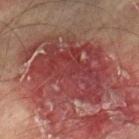biopsy status = total-body-photography surveillance lesion; no biopsy | lighting = cross-polarized | image-analysis metrics = a lesion area of about 60 mm², a shape eccentricity near 0.7, and two-axis asymmetry of about 0.25; a border-irregularity index near 5.5/10, internal color variation of about 6.5 on a 0–10 scale, and a peripheral color-asymmetry measure near 2; a detector confidence of about 85 out of 100 that the crop contains a lesion | imaging modality = ~15 mm crop, total-body skin-cancer survey | body site = the leg | patient = male, roughly 75 years of age.